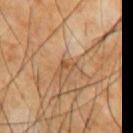Context:
A region of skin cropped from a whole-body photographic capture, roughly 15 mm wide. The total-body-photography lesion software estimated a border-irregularity rating of about 6/10, internal color variation of about 0 on a 0–10 scale, and peripheral color asymmetry of about 0. It also reported a classifier nevus-likeness of about 0/100 and a lesion-detection confidence of about 95/100. The recorded lesion diameter is about 2.5 mm. The lesion is located on the chest. A male subject aged around 65.